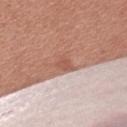The lesion was photographed on a routine skin check and not biopsied; there is no pathology result. A 15 mm crop from a total-body photograph taken for skin-cancer surveillance. The lesion is on the arm. A female subject, approximately 55 years of age.